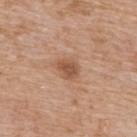No biopsy was performed on this lesion — it was imaged during a full skin examination and was not determined to be concerning. A close-up tile cropped from a whole-body skin photograph, about 15 mm across. On the upper back. The subject is a male aged around 60.